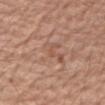- subject — male, approximately 70 years of age
- image source — ~15 mm crop, total-body skin-cancer survey
- body site — the right forearm
- lighting — white-light illumination
- image-analysis metrics — an average lesion color of about L≈53 a*≈22 b*≈30 (CIELAB), roughly 5 lightness units darker than nearby skin, and a normalized border contrast of about 4; border irregularity of about 3 on a 0–10 scale, a color-variation rating of about 0/10, and radial color variation of about 0; a nevus-likeness score of about 0/100 and a lesion-detection confidence of about 100/100
- size — ≈1 mm